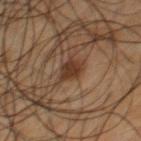Clinical impression:
Recorded during total-body skin imaging; not selected for excision or biopsy.
Background:
A male subject, in their mid- to late 50s. Cropped from a whole-body photographic skin survey; the tile spans about 15 mm. An algorithmic analysis of the crop reported an area of roughly 4 mm² and a shape-asymmetry score of about 0.35 (0 = symmetric). The analysis additionally found an average lesion color of about L≈32 a*≈17 b*≈26 (CIELAB) and a lesion-to-skin contrast of about 10 (normalized; higher = more distinct). The software also gave border irregularity of about 3 on a 0–10 scale and peripheral color asymmetry of about 0.5. From the chest.